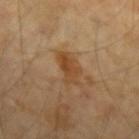notes=no biopsy performed (imaged during a skin exam)
automated metrics=a lesion area of about 8 mm² and an eccentricity of roughly 0.85; roughly 8 lightness units darker than nearby skin; border irregularity of about 4 on a 0–10 scale and a peripheral color-asymmetry measure near 1.5; a classifier nevus-likeness of about 75/100 and a detector confidence of about 100 out of 100 that the crop contains a lesion
subject=male, in their mid- to late 40s
lighting=cross-polarized illumination
acquisition=total-body-photography crop, ~15 mm field of view
diameter=≈4.5 mm
body site=the left forearm